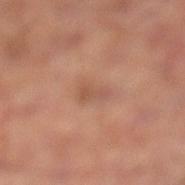Findings:
– follow-up: total-body-photography surveillance lesion; no biopsy
– imaging modality: ~15 mm crop, total-body skin-cancer survey
– subject: male, aged 63–67
– size: ~3 mm (longest diameter)
– body site: the left thigh
– lighting: cross-polarized illumination